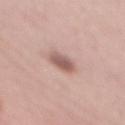This lesion was catalogued during total-body skin photography and was not selected for biopsy. The total-body-photography lesion software estimated an area of roughly 5.5 mm² and an eccentricity of roughly 0.85. It also reported a mean CIELAB color near L≈58 a*≈20 b*≈23, a lesion–skin lightness drop of about 13, and a lesion-to-skin contrast of about 8 (normalized; higher = more distinct). Cropped from a whole-body photographic skin survey; the tile spans about 15 mm. The tile uses white-light illumination. From the mid back. A female patient, roughly 60 years of age.